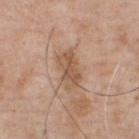Captured during whole-body skin photography for melanoma surveillance; the lesion was not biopsied. A male patient, in their 50s. Located on the chest. Imaged with white-light lighting. Measured at roughly 4.5 mm in maximum diameter. A roughly 15 mm field-of-view crop from a total-body skin photograph. Automated tile analysis of the lesion measured a lesion–skin lightness drop of about 9 and a lesion-to-skin contrast of about 7 (normalized; higher = more distinct). It also reported a border-irregularity rating of about 2.5/10 and a within-lesion color-variation index near 4/10. And it measured an automated nevus-likeness rating near 0 out of 100 and a detector confidence of about 100 out of 100 that the crop contains a lesion.